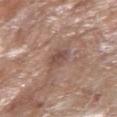Q: Was this lesion biopsied?
A: imaged on a skin check; not biopsied
Q: Patient demographics?
A: female, aged 73 to 77
Q: Automated lesion metrics?
A: an area of roughly 5 mm², an outline eccentricity of about 0.75 (0 = round, 1 = elongated), and a symmetry-axis asymmetry near 0.3
Q: How was this image acquired?
A: 15 mm crop, total-body photography
Q: Where on the body is the lesion?
A: the right forearm
Q: How large is the lesion?
A: about 3 mm